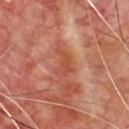follow-up=imaged on a skin check; not biopsied
size=about 3 mm
tile lighting=cross-polarized illumination
subject=male, aged approximately 70
body site=the chest
image source=total-body-photography crop, ~15 mm field of view
automated metrics=a mean CIELAB color near L≈49 a*≈30 b*≈33, a lesion–skin lightness drop of about 8, and a normalized border contrast of about 6; border irregularity of about 6 on a 0–10 scale, a color-variation rating of about 2/10, and a peripheral color-asymmetry measure near 0.5